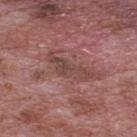Notes:
- illumination — white-light illumination
- lesion size — about 5.5 mm
- imaging modality — ~15 mm tile from a whole-body skin photo
- location — the back
- subject — male, aged around 70
- image-analysis metrics — a lesion area of about 7 mm² and a shape eccentricity near 0.95; an average lesion color of about L≈44 a*≈21 b*≈22 (CIELAB) and a normalized lesion–skin contrast near 6